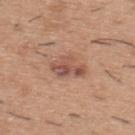Part of a total-body skin-imaging series; this lesion was reviewed on a skin check and was not flagged for biopsy. On the upper back. The lesion's longest dimension is about 4 mm. This is a white-light tile. A male patient, approximately 40 years of age. A 15 mm crop from a total-body photograph taken for skin-cancer surveillance.